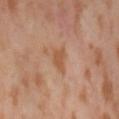Impression: The lesion was photographed on a routine skin check and not biopsied; there is no pathology result. Context: The lesion is located on the lower back. The total-body-photography lesion software estimated an area of roughly 4 mm² and an outline eccentricity of about 0.65 (0 = round, 1 = elongated). It also reported a border-irregularity index near 4/10, internal color variation of about 1 on a 0–10 scale, and peripheral color asymmetry of about 0.5. A lesion tile, about 15 mm wide, cut from a 3D total-body photograph. A female patient, approximately 55 years of age. Captured under cross-polarized illumination. Longest diameter approximately 2.5 mm.